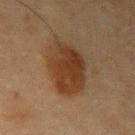Recorded during total-body skin imaging; not selected for excision or biopsy. Cropped from a total-body skin-imaging series; the visible field is about 15 mm. From the right upper arm. The subject is a male roughly 65 years of age. Approximately 6 mm at its widest. The tile uses cross-polarized illumination.